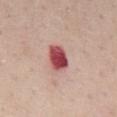notes: imaged on a skin check; not biopsied | TBP lesion metrics: a lesion area of about 7.5 mm², a shape eccentricity near 0.65, and two-axis asymmetry of about 0.25; a mean CIELAB color near L≈50 a*≈33 b*≈22, a lesion–skin lightness drop of about 20, and a lesion-to-skin contrast of about 13 (normalized; higher = more distinct); a border-irregularity index near 2/10 and a peripheral color-asymmetry measure near 1.5; a classifier nevus-likeness of about 0/100 and a detector confidence of about 100 out of 100 that the crop contains a lesion | imaging modality: total-body-photography crop, ~15 mm field of view | illumination: white-light | patient: male, in their mid-50s | lesion size: ≈3.5 mm | location: the mid back.